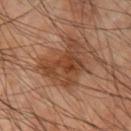  biopsy_status: not biopsied; imaged during a skin examination
  image:
    source: total-body photography crop
    field_of_view_mm: 15
  site: arm
  patient:
    sex: male
    age_approx: 60